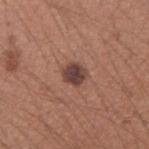  biopsy_status: not biopsied; imaged during a skin examination
  image:
    source: total-body photography crop
    field_of_view_mm: 15
  patient:
    sex: male
    age_approx: 35
  lighting: white-light
  site: arm
  lesion_size:
    long_diameter_mm_approx: 2.5
  automated_metrics:
    area_mm2_approx: 5.0
    eccentricity: 0.35
    cielab_L: 41
    cielab_a: 19
    cielab_b: 22
    vs_skin_darker_L: 13.0